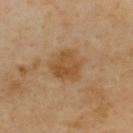Acquisition and patient details:
The recorded lesion diameter is about 4 mm. This image is a 15 mm lesion crop taken from a total-body photograph. On the upper back. A male patient aged 53 to 57.